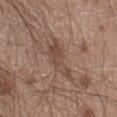Imaged during a routine full-body skin examination; the lesion was not biopsied and no histopathology is available.
Captured under white-light illumination.
The lesion's longest dimension is about 5.5 mm.
Automated tile analysis of the lesion measured an area of roughly 8.5 mm², an outline eccentricity of about 0.9 (0 = round, 1 = elongated), and a shape-asymmetry score of about 0.4 (0 = symmetric). It also reported a color-variation rating of about 3/10 and radial color variation of about 1. And it measured a classifier nevus-likeness of about 0/100 and a detector confidence of about 65 out of 100 that the crop contains a lesion.
The patient is a male aged around 20.
On the left lower leg.
Cropped from a whole-body photographic skin survey; the tile spans about 15 mm.